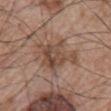Automated image analysis of the tile measured a border-irregularity rating of about 8.5/10 and a within-lesion color-variation index near 5.5/10. The analysis additionally found a detector confidence of about 55 out of 100 that the crop contains a lesion. A 15 mm crop from a total-body photograph taken for skin-cancer surveillance. Longest diameter approximately 6 mm. Captured under white-light illumination. A male patient, aged 63 to 67. The lesion is on the chest.